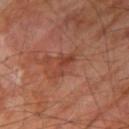workup = total-body-photography surveillance lesion; no biopsy | lighting = cross-polarized illumination | body site = the right thigh | automated lesion analysis = a lesion color around L≈41 a*≈26 b*≈30 in CIELAB, about 7 CIELAB-L* units darker than the surrounding skin, and a normalized border contrast of about 6; internal color variation of about 0.5 on a 0–10 scale; an automated nevus-likeness rating near 0 out of 100 and a lesion-detection confidence of about 100/100 | patient = male, aged approximately 70 | imaging modality = total-body-photography crop, ~15 mm field of view | lesion diameter = ≈3.5 mm.